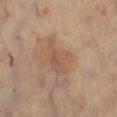Q: Was this lesion biopsied?
A: no biopsy performed (imaged during a skin exam)
Q: Automated lesion metrics?
A: an area of roughly 8.5 mm², an outline eccentricity of about 0.8 (0 = round, 1 = elongated), and two-axis asymmetry of about 0.45; a lesion–skin lightness drop of about 7 and a lesion-to-skin contrast of about 5 (normalized; higher = more distinct); border irregularity of about 5 on a 0–10 scale, a within-lesion color-variation index near 4/10, and radial color variation of about 1.5; a classifier nevus-likeness of about 0/100
Q: What are the patient's age and sex?
A: approximately 60 years of age
Q: What lighting was used for the tile?
A: cross-polarized illumination
Q: What is the imaging modality?
A: 15 mm crop, total-body photography
Q: Where on the body is the lesion?
A: the leg